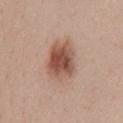Clinical impression:
Recorded during total-body skin imaging; not selected for excision or biopsy.
Image and clinical context:
A female patient aged approximately 45. Cropped from a total-body skin-imaging series; the visible field is about 15 mm. Imaged with white-light lighting. From the chest. The recorded lesion diameter is about 5 mm.